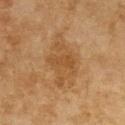The lesion was photographed on a routine skin check and not biopsied; there is no pathology result. Automated tile analysis of the lesion measured border irregularity of about 4.5 on a 0–10 scale, internal color variation of about 2.5 on a 0–10 scale, and a peripheral color-asymmetry measure near 0.5. On the front of the torso. The recorded lesion diameter is about 6.5 mm. Imaged with cross-polarized lighting. A female patient in their 60s. A lesion tile, about 15 mm wide, cut from a 3D total-body photograph.